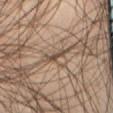follow-up: imaged on a skin check; not biopsied
diameter: about 2.5 mm
image source: ~15 mm crop, total-body skin-cancer survey
anatomic site: the abdomen
image-analysis metrics: a shape eccentricity near 0.95 and a shape-asymmetry score of about 0.3 (0 = symmetric); border irregularity of about 3.5 on a 0–10 scale, a within-lesion color-variation index near 0/10, and a peripheral color-asymmetry measure near 0
subject: male, in their mid- to late 50s